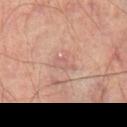biopsy_status: not biopsied; imaged during a skin examination
lesion_size:
  long_diameter_mm_approx: 3.0
image:
  source: total-body photography crop
  field_of_view_mm: 15
site: left thigh
lighting: cross-polarized
patient:
  sex: male
  age_approx: 70
automated_metrics:
  eccentricity: 0.7
  shape_asymmetry: 0.5
  cielab_L: 58
  cielab_a: 21
  cielab_b: 24
  vs_skin_darker_L: 6.0
  vs_skin_contrast_norm: 4.5
  border_irregularity_0_10: 5.5
  color_variation_0_10: 1.5
  peripheral_color_asymmetry: 0.5